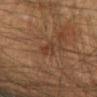  biopsy_status: not biopsied; imaged during a skin examination
  lesion_size:
    long_diameter_mm_approx: 2.5
  site: left forearm
  automated_metrics:
    area_mm2_approx: 3.0
    eccentricity: 0.85
    shape_asymmetry: 0.3
    cielab_L: 33
    cielab_a: 18
    cielab_b: 26
    vs_skin_darker_L: 6.0
    vs_skin_contrast_norm: 6.0
    border_irregularity_0_10: 3.5
    color_variation_0_10: 0.0
    peripheral_color_asymmetry: 0.0
  image:
    source: total-body photography crop
    field_of_view_mm: 15
  patient:
    sex: male
    age_approx: 40